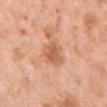The lesion was photographed on a routine skin check and not biopsied; there is no pathology result. The lesion-visualizer software estimated an average lesion color of about L≈61 a*≈24 b*≈35 (CIELAB), about 10 CIELAB-L* units darker than the surrounding skin, and a normalized border contrast of about 6.5. And it measured radial color variation of about 1. The analysis additionally found a lesion-detection confidence of about 100/100. Measured at roughly 3.5 mm in maximum diameter. A female subject roughly 50 years of age. Cropped from a whole-body photographic skin survey; the tile spans about 15 mm. The tile uses white-light illumination. The lesion is located on the chest.